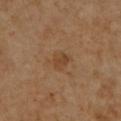Q: Was this lesion biopsied?
A: catalogued during a skin exam; not biopsied
Q: What is the anatomic site?
A: the right lower leg
Q: What are the patient's age and sex?
A: female, about 50 years old
Q: What is the imaging modality?
A: ~15 mm tile from a whole-body skin photo
Q: What did automated image analysis measure?
A: an area of roughly 4.5 mm², an eccentricity of roughly 0.7, and a shape-asymmetry score of about 0.2 (0 = symmetric); border irregularity of about 2 on a 0–10 scale, a within-lesion color-variation index near 1.5/10, and peripheral color asymmetry of about 0.5; a nevus-likeness score of about 5/100 and a lesion-detection confidence of about 100/100